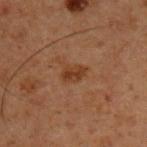Captured during whole-body skin photography for melanoma surveillance; the lesion was not biopsied. Longest diameter approximately 2.5 mm. A male patient, aged 58 to 62. Imaged with cross-polarized lighting. This image is a 15 mm lesion crop taken from a total-body photograph. The lesion is located on the right upper arm.